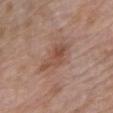This lesion was catalogued during total-body skin photography and was not selected for biopsy.
Longest diameter approximately 5 mm.
The patient is a male roughly 70 years of age.
Captured under white-light illumination.
On the left upper arm.
A 15 mm crop from a total-body photograph taken for skin-cancer surveillance.